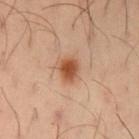{
  "biopsy_status": "not biopsied; imaged during a skin examination",
  "patient": {
    "sex": "male",
    "age_approx": 40
  },
  "lighting": "cross-polarized",
  "site": "left upper arm",
  "lesion_size": {
    "long_diameter_mm_approx": 3.0
  },
  "automated_metrics": {
    "eccentricity": 0.65,
    "shape_asymmetry": 0.2,
    "cielab_L": 51,
    "cielab_a": 25,
    "cielab_b": 34,
    "vs_skin_darker_L": 13.0,
    "vs_skin_contrast_norm": 10.0,
    "border_irregularity_0_10": 2.0,
    "color_variation_0_10": 3.0,
    "peripheral_color_asymmetry": 1.0,
    "nevus_likeness_0_100": 100,
    "lesion_detection_confidence_0_100": 100
  },
  "image": {
    "source": "total-body photography crop",
    "field_of_view_mm": 15
  }
}